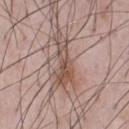Imaged during a routine full-body skin examination; the lesion was not biopsied and no histopathology is available.
This image is a 15 mm lesion crop taken from a total-body photograph.
About 5.5 mm across.
This is a white-light tile.
From the chest.
A male patient, approximately 50 years of age.